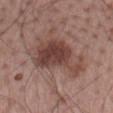biopsy_status: not biopsied; imaged during a skin examination
patient:
  sex: male
  age_approx: 70
image:
  source: total-body photography crop
  field_of_view_mm: 15
lesion_size:
  long_diameter_mm_approx: 7.5
automated_metrics:
  cielab_L: 42
  cielab_a: 20
  cielab_b: 23
  vs_skin_darker_L: 12.0
  vs_skin_contrast_norm: 9.0
  nevus_likeness_0_100: 70
  lesion_detection_confidence_0_100: 100
site: mid back
lighting: white-light